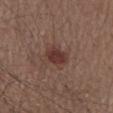The lesion is on the chest. A close-up tile cropped from a whole-body skin photograph, about 15 mm across. A male patient, aged 53 to 57. This is a white-light tile. Longest diameter approximately 3 mm. The total-body-photography lesion software estimated an eccentricity of roughly 0.55 and two-axis asymmetry of about 0.2. The analysis additionally found about 9 CIELAB-L* units darker than the surrounding skin and a normalized lesion–skin contrast near 8.5. The analysis additionally found a border-irregularity index near 2/10, internal color variation of about 2 on a 0–10 scale, and peripheral color asymmetry of about 0.5. The analysis additionally found a nevus-likeness score of about 95/100 and a lesion-detection confidence of about 100/100.